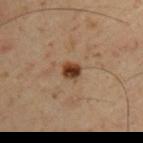<record>
  <biopsy_status>not biopsied; imaged during a skin examination</biopsy_status>
  <image>
    <source>total-body photography crop</source>
    <field_of_view_mm>15</field_of_view_mm>
  </image>
  <lesion_size>
    <long_diameter_mm_approx>2.0</long_diameter_mm_approx>
  </lesion_size>
  <automated_metrics>
    <area_mm2_approx>3.5</area_mm2_approx>
    <eccentricity>0.45</eccentricity>
    <shape_asymmetry>0.2</shape_asymmetry>
    <border_irregularity_0_10>1.5</border_irregularity_0_10>
    <color_variation_0_10>5.0</color_variation_0_10>
    <peripheral_color_asymmetry>1.5</peripheral_color_asymmetry>
    <nevus_likeness_0_100>100</nevus_likeness_0_100>
    <lesion_detection_confidence_0_100>100</lesion_detection_confidence_0_100>
  </automated_metrics>
  <patient>
    <sex>male</sex>
    <age_approx>50</age_approx>
  </patient>
  <site>arm</site>
</record>